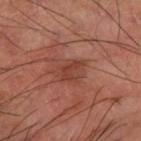automated metrics — a lesion area of about 5.5 mm² and an outline eccentricity of about 0.75 (0 = round, 1 = elongated); a mean CIELAB color near L≈42 a*≈27 b*≈28, about 7 CIELAB-L* units darker than the surrounding skin, and a normalized lesion–skin contrast near 6; a border-irregularity rating of about 3/10, a color-variation rating of about 2/10, and peripheral color asymmetry of about 0.5; an automated nevus-likeness rating near 0 out of 100 and a detector confidence of about 100 out of 100 that the crop contains a lesion | imaging modality — ~15 mm tile from a whole-body skin photo | patient — male, in their mid- to late 60s | lesion size — ≈3.5 mm | illumination — cross-polarized illumination | anatomic site — the left forearm.